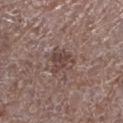| key | value |
|---|---|
| biopsy status | imaged on a skin check; not biopsied |
| subject | male, approximately 70 years of age |
| automated metrics | a lesion area of about 7 mm², an eccentricity of roughly 0.55, and a symmetry-axis asymmetry near 0.35; a color-variation rating of about 4.5/10 and a peripheral color-asymmetry measure near 2; a classifier nevus-likeness of about 0/100 and a detector confidence of about 100 out of 100 that the crop contains a lesion |
| image source | ~15 mm tile from a whole-body skin photo |
| body site | the left lower leg |